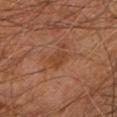| key | value |
|---|---|
| workup | catalogued during a skin exam; not biopsied |
| patient | male, aged approximately 60 |
| lesion size | ~2.5 mm (longest diameter) |
| illumination | cross-polarized |
| body site | the right leg |
| acquisition | ~15 mm crop, total-body skin-cancer survey |